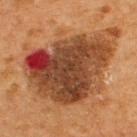- biopsy status — imaged on a skin check; not biopsied
- subject — male, aged around 65
- illumination — cross-polarized
- anatomic site — the back
- size — ≈10 mm
- image-analysis metrics — a border-irregularity index near 4/10, internal color variation of about 10 on a 0–10 scale, and peripheral color asymmetry of about 4
- imaging modality — ~15 mm crop, total-body skin-cancer survey